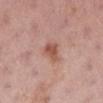notes = total-body-photography surveillance lesion; no biopsy | image = ~15 mm crop, total-body skin-cancer survey | site = the left lower leg | subject = female, aged 53 to 57.